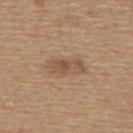notes: catalogued during a skin exam; not biopsied
acquisition: total-body-photography crop, ~15 mm field of view
location: the upper back
patient: male, aged around 65
size: ≈4 mm